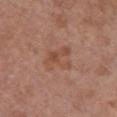Captured during whole-body skin photography for melanoma surveillance; the lesion was not biopsied. Located on the chest. Cropped from a total-body skin-imaging series; the visible field is about 15 mm. Longest diameter approximately 3.5 mm. Automated image analysis of the tile measured an average lesion color of about L≈49 a*≈22 b*≈30 (CIELAB), about 6 CIELAB-L* units darker than the surrounding skin, and a normalized border contrast of about 5.5. The software also gave a color-variation rating of about 4/10 and a peripheral color-asymmetry measure near 1.5. The analysis additionally found a detector confidence of about 100 out of 100 that the crop contains a lesion. A female subject, roughly 75 years of age. This is a white-light tile.